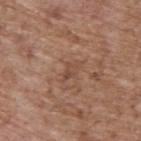* follow-up: catalogued during a skin exam; not biopsied
* image-analysis metrics: a within-lesion color-variation index near 1.5/10 and peripheral color asymmetry of about 0.5
* imaging modality: ~15 mm crop, total-body skin-cancer survey
* lesion size: ≈3 mm
* subject: male, in their 70s
* lighting: white-light illumination
* body site: the upper back